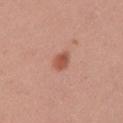Captured during whole-body skin photography for melanoma surveillance; the lesion was not biopsied. A female subject, in their mid- to late 30s. The lesion is on the arm. A region of skin cropped from a whole-body photographic capture, roughly 15 mm wide. The tile uses white-light illumination. An algorithmic analysis of the crop reported an average lesion color of about L≈54 a*≈26 b*≈31 (CIELAB) and a lesion-to-skin contrast of about 7.5 (normalized; higher = more distinct). The analysis additionally found an automated nevus-likeness rating near 95 out of 100 and a lesion-detection confidence of about 100/100. The recorded lesion diameter is about 2.5 mm.